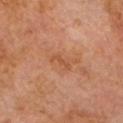<tbp_lesion>
<biopsy_status>not biopsied; imaged during a skin examination</biopsy_status>
<lesion_size>
  <long_diameter_mm_approx>2.5</long_diameter_mm_approx>
</lesion_size>
<patient>
  <sex>male</sex>
  <age_approx>60</age_approx>
</patient>
<lighting>cross-polarized</lighting>
<site>head or neck</site>
<image>
  <source>total-body photography crop</source>
  <field_of_view_mm>15</field_of_view_mm>
</image>
</tbp_lesion>